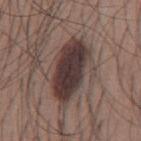Clinical impression: The lesion was photographed on a routine skin check and not biopsied; there is no pathology result. Background: A close-up tile cropped from a whole-body skin photograph, about 15 mm across. Longest diameter approximately 8 mm. The tile uses white-light illumination. On the mid back. A male patient roughly 35 years of age.